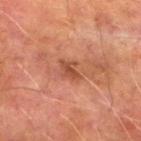Case summary:
– subject — male, roughly 75 years of age
– TBP lesion metrics — a lesion color around L≈40 a*≈24 b*≈29 in CIELAB and a normalized lesion–skin contrast near 6.5; a nevus-likeness score of about 0/100 and a detector confidence of about 100 out of 100 that the crop contains a lesion
– acquisition — ~15 mm crop, total-body skin-cancer survey
– lesion diameter — about 2.5 mm
– tile lighting — cross-polarized
– body site — the left lower leg